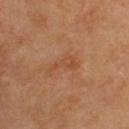The lesion was tiled from a total-body skin photograph and was not biopsied. The patient is a male aged 68–72. A 15 mm close-up tile from a total-body photography series done for melanoma screening. Captured under cross-polarized illumination. Measured at roughly 4 mm in maximum diameter. From the upper back. Automated image analysis of the tile measured an outline eccentricity of about 0.95 (0 = round, 1 = elongated). It also reported a border-irregularity rating of about 5.5/10, a color-variation rating of about 2.5/10, and peripheral color asymmetry of about 0.5.